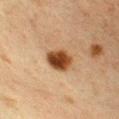image = ~15 mm crop, total-body skin-cancer survey; patient = female, aged approximately 40; tile lighting = cross-polarized illumination; site = the chest.